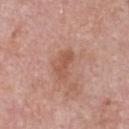follow-up = imaged on a skin check; not biopsied
TBP lesion metrics = a classifier nevus-likeness of about 0/100 and lesion-presence confidence of about 100/100
imaging modality = 15 mm crop, total-body photography
lighting = white-light
patient = male, about 55 years old
site = the chest
size = ~3.5 mm (longest diameter)